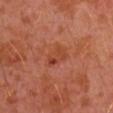Captured during whole-body skin photography for melanoma surveillance; the lesion was not biopsied. Located on the left arm. Longest diameter approximately 3 mm. This is a cross-polarized tile. A roughly 15 mm field-of-view crop from a total-body skin photograph. A male patient approximately 30 years of age. Automated tile analysis of the lesion measured an average lesion color of about L≈43 a*≈29 b*≈34 (CIELAB) and roughly 6 lightness units darker than nearby skin. The software also gave a border-irregularity index near 2.5/10, a within-lesion color-variation index near 6/10, and peripheral color asymmetry of about 2. The analysis additionally found a classifier nevus-likeness of about 0/100 and a lesion-detection confidence of about 100/100.